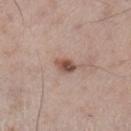Findings:
– follow-up: total-body-photography surveillance lesion; no biopsy
– patient: male, about 60 years old
– size: about 2.5 mm
– lighting: white-light
– site: the leg
– automated metrics: an area of roughly 4 mm², an outline eccentricity of about 0.8 (0 = round, 1 = elongated), and a symmetry-axis asymmetry near 0.25; a mean CIELAB color near L≈51 a*≈20 b*≈25, a lesion–skin lightness drop of about 13, and a normalized border contrast of about 9.5
– imaging modality: total-body-photography crop, ~15 mm field of view